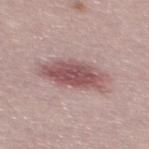Recorded during total-body skin imaging; not selected for excision or biopsy.
This image is a 15 mm lesion crop taken from a total-body photograph.
A male subject, aged 28–32.